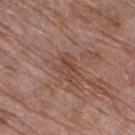biopsy status: catalogued during a skin exam; not biopsied
patient: female, aged 68 to 72
size: about 3 mm
image-analysis metrics: an area of roughly 4 mm², a shape eccentricity near 0.85, and a shape-asymmetry score of about 0.35 (0 = symmetric); a detector confidence of about 95 out of 100 that the crop contains a lesion
site: the right thigh
image: 15 mm crop, total-body photography